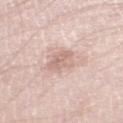Background:
A lesion tile, about 15 mm wide, cut from a 3D total-body photograph. A male patient, approximately 50 years of age. This is a white-light tile. The lesion is located on the left lower leg. The lesion's longest dimension is about 3.5 mm.